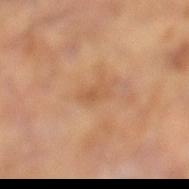Imaged during a routine full-body skin examination; the lesion was not biopsied and no histopathology is available. A male subject in their mid- to late 60s. Captured under cross-polarized illumination. Located on the left lower leg. Longest diameter approximately 2.5 mm. Cropped from a whole-body photographic skin survey; the tile spans about 15 mm.